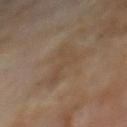| feature | finding |
|---|---|
| follow-up | catalogued during a skin exam; not biopsied |
| lesion size | ≈5.5 mm |
| anatomic site | the back |
| image | ~15 mm tile from a whole-body skin photo |
| patient | male, roughly 70 years of age |